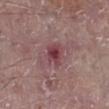Assessment:
Part of a total-body skin-imaging series; this lesion was reviewed on a skin check and was not flagged for biopsy.
Context:
Located on the leg. A roughly 15 mm field-of-view crop from a total-body skin photograph. A male patient aged 73 to 77. Approximately 2.5 mm at its widest. Imaged with white-light lighting.